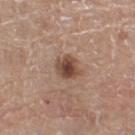<record>
  <biopsy_status>not biopsied; imaged during a skin examination</biopsy_status>
  <image>
    <source>total-body photography crop</source>
    <field_of_view_mm>15</field_of_view_mm>
  </image>
  <lighting>white-light</lighting>
  <automated_metrics>
    <area_mm2_approx>6.5</area_mm2_approx>
    <cielab_L>46</cielab_L>
    <cielab_a>19</cielab_a>
    <cielab_b>27</cielab_b>
    <vs_skin_darker_L>13.0</vs_skin_darker_L>
    <vs_skin_contrast_norm>9.5</vs_skin_contrast_norm>
  </automated_metrics>
  <patient>
    <sex>female</sex>
    <age_approx>75</age_approx>
  </patient>
  <site>left thigh</site>
</record>